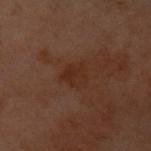Recorded during total-body skin imaging; not selected for excision or biopsy. From the front of the torso. Longest diameter approximately 3 mm. The patient is a female aged 58–62. A 15 mm close-up tile from a total-body photography series done for melanoma screening.